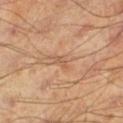Recorded during total-body skin imaging; not selected for excision or biopsy.
Cropped from a total-body skin-imaging series; the visible field is about 15 mm.
The subject is a male roughly 45 years of age.
On the left lower leg.